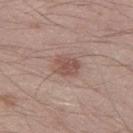Impression: This lesion was catalogued during total-body skin photography and was not selected for biopsy. Acquisition and patient details: Imaged with white-light lighting. A male patient in their mid- to late 30s. Approximately 3.5 mm at its widest. The lesion is on the left thigh. The lesion-visualizer software estimated a mean CIELAB color near L≈52 a*≈19 b*≈24, about 9 CIELAB-L* units darker than the surrounding skin, and a normalized lesion–skin contrast near 7. It also reported border irregularity of about 2 on a 0–10 scale, a color-variation rating of about 2.5/10, and peripheral color asymmetry of about 1. And it measured a nevus-likeness score of about 65/100 and lesion-presence confidence of about 100/100. A 15 mm crop from a total-body photograph taken for skin-cancer surveillance.